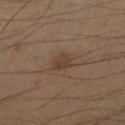workup: catalogued during a skin exam; not biopsied
patient: male, aged 38–42
image source: ~15 mm tile from a whole-body skin photo
site: the right forearm